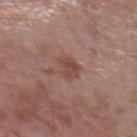Recorded during total-body skin imaging; not selected for excision or biopsy.
A lesion tile, about 15 mm wide, cut from a 3D total-body photograph.
An algorithmic analysis of the crop reported a lesion area of about 5 mm², a shape eccentricity near 0.35, and two-axis asymmetry of about 0.35. The analysis additionally found a lesion color around L≈46 a*≈21 b*≈25 in CIELAB and a normalized lesion–skin contrast near 6.5.
A male subject approximately 40 years of age.
Imaged with white-light lighting.
Located on the arm.
About 2.5 mm across.